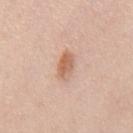Q: Was this lesion biopsied?
A: total-body-photography surveillance lesion; no biopsy
Q: Patient demographics?
A: male, approximately 60 years of age
Q: Illumination type?
A: white-light illumination
Q: Lesion size?
A: about 3.5 mm
Q: What is the imaging modality?
A: total-body-photography crop, ~15 mm field of view
Q: Lesion location?
A: the back